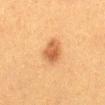Clinical impression: This lesion was catalogued during total-body skin photography and was not selected for biopsy. Clinical summary: Captured under cross-polarized illumination. The recorded lesion diameter is about 3.5 mm. A region of skin cropped from a whole-body photographic capture, roughly 15 mm wide. A female patient in their 40s. On the abdomen.